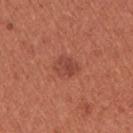  biopsy_status: not biopsied; imaged during a skin examination
  site: arm
  lighting: white-light
  automated_metrics:
    area_mm2_approx: 5.0
    eccentricity: 0.65
    shape_asymmetry: 0.3
    border_irregularity_0_10: 2.5
    color_variation_0_10: 3.0
    peripheral_color_asymmetry: 1.0
    lesion_detection_confidence_0_100: 100
  image:
    source: total-body photography crop
    field_of_view_mm: 15
  patient:
    sex: female
    age_approx: 35
  lesion_size:
    long_diameter_mm_approx: 2.5